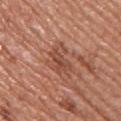image-analysis metrics — an area of roughly 8.5 mm², an outline eccentricity of about 0.65 (0 = round, 1 = elongated), and a symmetry-axis asymmetry near 0.4; imaging modality — total-body-photography crop, ~15 mm field of view; subject — male, approximately 55 years of age; location — the upper back.